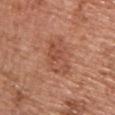Clinical impression:
This lesion was catalogued during total-body skin photography and was not selected for biopsy.
Background:
A male patient in their mid- to late 50s. A 15 mm crop from a total-body photograph taken for skin-cancer surveillance. The recorded lesion diameter is about 5 mm. Captured under white-light illumination. From the chest.